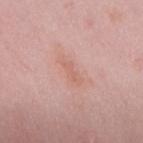Q: Was this lesion biopsied?
A: catalogued during a skin exam; not biopsied
Q: What is the imaging modality?
A: ~15 mm tile from a whole-body skin photo
Q: What are the patient's age and sex?
A: female, about 50 years old
Q: How large is the lesion?
A: ≈3.5 mm
Q: What is the anatomic site?
A: the upper back
Q: What did automated image analysis measure?
A: a lesion area of about 4 mm² and a symmetry-axis asymmetry near 0.35